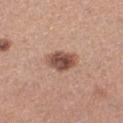Imaged during a routine full-body skin examination; the lesion was not biopsied and no histopathology is available.
A female subject, aged approximately 30.
Located on the leg.
Captured under white-light illumination.
Cropped from a total-body skin-imaging series; the visible field is about 15 mm.
The recorded lesion diameter is about 3.5 mm.